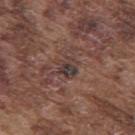Impression: The lesion was tiled from a total-body skin photograph and was not biopsied. Background: A roughly 15 mm field-of-view crop from a total-body skin photograph. The lesion is located on the upper back. Longest diameter approximately 2.5 mm. The subject is a male aged around 75. Automated tile analysis of the lesion measured an outline eccentricity of about 0.4 (0 = round, 1 = elongated) and a symmetry-axis asymmetry near 0.2. And it measured an average lesion color of about L≈37 a*≈15 b*≈18 (CIELAB), a lesion–skin lightness drop of about 8, and a normalized border contrast of about 8.5. The analysis additionally found an automated nevus-likeness rating near 0 out of 100 and a lesion-detection confidence of about 85/100. This is a white-light tile.